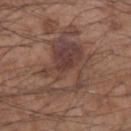| key | value |
|---|---|
| biopsy status | no biopsy performed (imaged during a skin exam) |
| illumination | white-light illumination |
| acquisition | ~15 mm tile from a whole-body skin photo |
| patient | male, in their mid-50s |
| body site | the left forearm |
| lesion size | ≈9.5 mm |